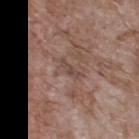  biopsy_status: not biopsied; imaged during a skin examination
  image:
    source: total-body photography crop
    field_of_view_mm: 15
  lesion_size:
    long_diameter_mm_approx: 3.0
  site: upper back
  automated_metrics:
    eccentricity: 0.95
    shape_asymmetry: 0.4
    cielab_L: 45
    cielab_a: 18
    cielab_b: 22
    vs_skin_darker_L: 8.0
    vs_skin_contrast_norm: 6.0
    border_irregularity_0_10: 4.5
  patient:
    sex: male
    age_approx: 70